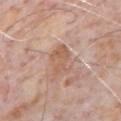Q: Is there a histopathology result?
A: catalogued during a skin exam; not biopsied
Q: What kind of image is this?
A: ~15 mm tile from a whole-body skin photo
Q: Where on the body is the lesion?
A: the chest
Q: What is the lesion's diameter?
A: about 4.5 mm
Q: What are the patient's age and sex?
A: male, about 80 years old
Q: How was the tile lit?
A: white-light illumination
Q: Automated lesion metrics?
A: a lesion area of about 7.5 mm², an outline eccentricity of about 0.85 (0 = round, 1 = elongated), and a shape-asymmetry score of about 0.4 (0 = symmetric); a mean CIELAB color near L≈59 a*≈20 b*≈30 and a lesion–skin lightness drop of about 8; a border-irregularity rating of about 4/10 and a peripheral color-asymmetry measure near 1; a classifier nevus-likeness of about 0/100 and a detector confidence of about 95 out of 100 that the crop contains a lesion